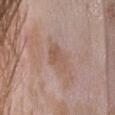Assessment:
Part of a total-body skin-imaging series; this lesion was reviewed on a skin check and was not flagged for biopsy.
Context:
A region of skin cropped from a whole-body photographic capture, roughly 15 mm wide. Located on the head or neck. The patient is a female aged approximately 25.